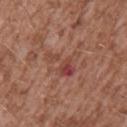Notes:
* notes — total-body-photography surveillance lesion; no biopsy
* patient — male, aged 43 to 47
* body site — the arm
* imaging modality — ~15 mm crop, total-body skin-cancer survey
* lesion size — ≈4 mm
* tile lighting — white-light illumination